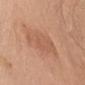The lesion was photographed on a routine skin check and not biopsied; there is no pathology result. The lesion is on the chest. The patient is a male aged 73–77. A 15 mm crop from a total-body photograph taken for skin-cancer surveillance.